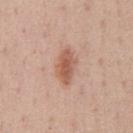Imaged during a routine full-body skin examination; the lesion was not biopsied and no histopathology is available.
Automated tile analysis of the lesion measured border irregularity of about 3 on a 0–10 scale, a color-variation rating of about 3.5/10, and a peripheral color-asymmetry measure near 1. The software also gave a classifier nevus-likeness of about 85/100 and a lesion-detection confidence of about 100/100.
A male subject aged 38–42.
This is a white-light tile.
This image is a 15 mm lesion crop taken from a total-body photograph.
On the chest.
Longest diameter approximately 4 mm.